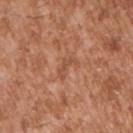Case summary:
- follow-up: imaged on a skin check; not biopsied
- subject: male, approximately 45 years of age
- body site: the right upper arm
- acquisition: ~15 mm crop, total-body skin-cancer survey